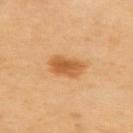– body site — the back
– patient — female, in their 70s
– acquisition — 15 mm crop, total-body photography
– illumination — cross-polarized illumination
– TBP lesion metrics — roughly 12 lightness units darker than nearby skin; a border-irregularity index near 2.5/10, internal color variation of about 3.5 on a 0–10 scale, and peripheral color asymmetry of about 1